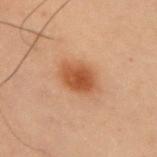Q: Is there a histopathology result?
A: imaged on a skin check; not biopsied
Q: What is the anatomic site?
A: the right upper arm
Q: What is the imaging modality?
A: total-body-photography crop, ~15 mm field of view
Q: Patient demographics?
A: male, about 55 years old